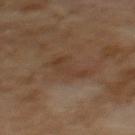Measured at roughly 4.5 mm in maximum diameter.
A male patient aged around 70.
A 15 mm close-up extracted from a 3D total-body photography capture.
The lesion is located on the mid back.